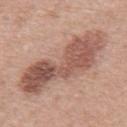Clinical impression: Captured during whole-body skin photography for melanoma surveillance; the lesion was not biopsied. Acquisition and patient details: From the mid back. The lesion's longest dimension is about 10.5 mm. The total-body-photography lesion software estimated an eccentricity of roughly 0.9 and a symmetry-axis asymmetry near 0.35. The analysis additionally found roughly 13 lightness units darker than nearby skin and a normalized border contrast of about 8.5. The patient is a male roughly 70 years of age. A lesion tile, about 15 mm wide, cut from a 3D total-body photograph. This is a white-light tile.